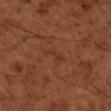Notes:
– workup: imaged on a skin check; not biopsied
– size: about 3 mm
– site: the right forearm
– image source: total-body-photography crop, ~15 mm field of view
– lighting: cross-polarized
– subject: male, about 60 years old
– automated lesion analysis: a lesion area of about 3 mm² and two-axis asymmetry of about 0.55